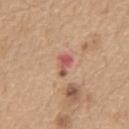Notes:
- follow-up · no biopsy performed (imaged during a skin exam)
- subject · male, aged 68 to 72
- location · the chest
- image · ~15 mm tile from a whole-body skin photo